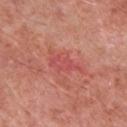biopsy status = catalogued during a skin exam; not biopsied | imaging modality = 15 mm crop, total-body photography | site = the front of the torso | patient = male, aged around 70.